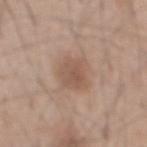Impression: Imaged during a routine full-body skin examination; the lesion was not biopsied and no histopathology is available. Acquisition and patient details: A region of skin cropped from a whole-body photographic capture, roughly 15 mm wide. On the mid back. A male patient roughly 45 years of age.